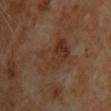| field | value |
|---|---|
| follow-up | catalogued during a skin exam; not biopsied |
| image source | 15 mm crop, total-body photography |
| automated lesion analysis | an area of roughly 16 mm², an eccentricity of roughly 0.85, and two-axis asymmetry of about 0.3; a detector confidence of about 100 out of 100 that the crop contains a lesion |
| lesion diameter | ~6 mm (longest diameter) |
| illumination | cross-polarized |
| subject | male, in their 70s |
| location | the upper back |